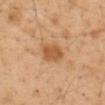biopsy status: total-body-photography surveillance lesion; no biopsy | image: 15 mm crop, total-body photography | diameter: ~3 mm (longest diameter) | patient: male, aged 48 to 52 | TBP lesion metrics: an area of roughly 6.5 mm², an outline eccentricity of about 0.65 (0 = round, 1 = elongated), and a shape-asymmetry score of about 0.25 (0 = symmetric); a border-irregularity index near 2/10, a within-lesion color-variation index near 3/10, and peripheral color asymmetry of about 1; an automated nevus-likeness rating near 85 out of 100 and a lesion-detection confidence of about 100/100 | anatomic site: the abdomen.